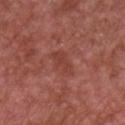The lesion was tiled from a total-body skin photograph and was not biopsied.
Measured at roughly 3.5 mm in maximum diameter.
The tile uses white-light illumination.
This image is a 15 mm lesion crop taken from a total-body photograph.
A male subject approximately 65 years of age.
From the front of the torso.